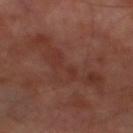Assessment: The lesion was tiled from a total-body skin photograph and was not biopsied. Context: The subject is a male aged 68–72. A 15 mm crop from a total-body photograph taken for skin-cancer surveillance. Imaged with cross-polarized lighting. About 8.5 mm across. The lesion is on the left thigh. Automated tile analysis of the lesion measured an average lesion color of about L≈33 a*≈22 b*≈24 (CIELAB) and a normalized lesion–skin contrast near 5. It also reported a border-irregularity index near 9.5/10, a color-variation rating of about 3.5/10, and radial color variation of about 1.